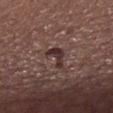Q: Was a biopsy performed?
A: no biopsy performed (imaged during a skin exam)
Q: Lesion size?
A: ≈3 mm
Q: Where on the body is the lesion?
A: the chest
Q: What did automated image analysis measure?
A: a footprint of about 4.5 mm², an eccentricity of roughly 0.8, and a symmetry-axis asymmetry near 0.6; an average lesion color of about L≈32 a*≈17 b*≈19 (CIELAB)
Q: What are the patient's age and sex?
A: male, approximately 55 years of age
Q: What kind of image is this?
A: ~15 mm tile from a whole-body skin photo